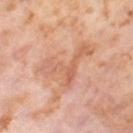{"biopsy_status": "not biopsied; imaged during a skin examination", "lesion_size": {"long_diameter_mm_approx": 6.5}, "patient": {"sex": "female", "age_approx": 55}, "site": "right thigh", "automated_metrics": {"cielab_L": 64, "cielab_a": 24, "cielab_b": 33, "vs_skin_darker_L": 8.0, "vs_skin_contrast_norm": 5.0, "border_irregularity_0_10": 9.5, "color_variation_0_10": 5.0, "peripheral_color_asymmetry": 1.5}, "lighting": "cross-polarized", "image": {"source": "total-body photography crop", "field_of_view_mm": 15}}